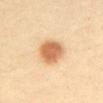follow-up: no biopsy performed (imaged during a skin exam)